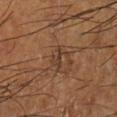Captured during whole-body skin photography for melanoma surveillance; the lesion was not biopsied. Measured at roughly 2.5 mm in maximum diameter. A male patient, in their mid- to late 60s. This is a cross-polarized tile. Located on the left lower leg. A 15 mm close-up extracted from a 3D total-body photography capture.